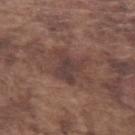Clinical impression: This lesion was catalogued during total-body skin photography and was not selected for biopsy. Image and clinical context: A roughly 15 mm field-of-view crop from a total-body skin photograph. This is a white-light tile. Measured at roughly 4 mm in maximum diameter. A male subject aged 73–77. The lesion is located on the left forearm.